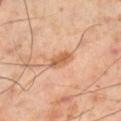No biopsy was performed on this lesion — it was imaged during a full skin examination and was not determined to be concerning. The total-body-photography lesion software estimated an automated nevus-likeness rating near 65 out of 100 and a detector confidence of about 100 out of 100 that the crop contains a lesion. Cropped from a total-body skin-imaging series; the visible field is about 15 mm. On the right lower leg. Longest diameter approximately 3.5 mm. The tile uses cross-polarized illumination. The patient is a male aged around 55.